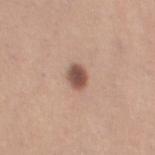Imaged during a routine full-body skin examination; the lesion was not biopsied and no histopathology is available. Cropped from a total-body skin-imaging series; the visible field is about 15 mm. A female patient in their 30s. Captured under white-light illumination. The lesion is on the left thigh. The total-body-photography lesion software estimated a footprint of about 5 mm², an outline eccentricity of about 0.65 (0 = round, 1 = elongated), and a shape-asymmetry score of about 0.2 (0 = symmetric).